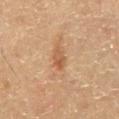Q: How large is the lesion?
A: ≈3 mm
Q: What kind of image is this?
A: total-body-photography crop, ~15 mm field of view
Q: Automated lesion metrics?
A: an average lesion color of about L≈49 a*≈19 b*≈32 (CIELAB), a lesion–skin lightness drop of about 8, and a lesion-to-skin contrast of about 6.5 (normalized; higher = more distinct); border irregularity of about 3.5 on a 0–10 scale, internal color variation of about 4.5 on a 0–10 scale, and radial color variation of about 1.5; a classifier nevus-likeness of about 0/100
Q: What lighting was used for the tile?
A: cross-polarized illumination
Q: What are the patient's age and sex?
A: male, aged around 60